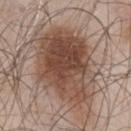– body site: the chest
– image: ~15 mm crop, total-body skin-cancer survey
– patient: male, aged 53 to 57